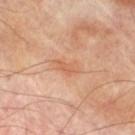Clinical impression:
No biopsy was performed on this lesion — it was imaged during a full skin examination and was not determined to be concerning.
Clinical summary:
Imaged with cross-polarized lighting. A lesion tile, about 15 mm wide, cut from a 3D total-body photograph. Located on the leg. The patient is a male in their 70s. Approximately 3 mm at its widest. An algorithmic analysis of the crop reported a lesion area of about 3.5 mm², an eccentricity of roughly 0.85, and two-axis asymmetry of about 0.4. And it measured about 7 CIELAB-L* units darker than the surrounding skin and a lesion-to-skin contrast of about 5.5 (normalized; higher = more distinct). It also reported an automated nevus-likeness rating near 0 out of 100 and lesion-presence confidence of about 100/100.